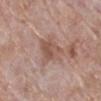Impression: This lesion was catalogued during total-body skin photography and was not selected for biopsy. Background: A 15 mm close-up tile from a total-body photography series done for melanoma screening. On the right lower leg. The subject is a female aged approximately 70.